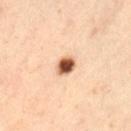* biopsy status — no biopsy performed (imaged during a skin exam)
* subject — male, roughly 65 years of age
* lesion diameter — about 2.5 mm
* location — the left thigh
* tile lighting — cross-polarized illumination
* imaging modality — total-body-photography crop, ~15 mm field of view